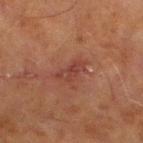workup = total-body-photography surveillance lesion; no biopsy | anatomic site = the right lower leg | image source = ~15 mm crop, total-body skin-cancer survey | patient = male, aged approximately 70 | size = ≈3.5 mm | illumination = cross-polarized.